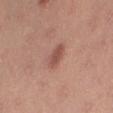This lesion was catalogued during total-body skin photography and was not selected for biopsy. A 15 mm crop from a total-body photograph taken for skin-cancer surveillance. The subject is a female aged 23 to 27. The lesion-visualizer software estimated an area of roughly 5.5 mm², an outline eccentricity of about 0.8 (0 = round, 1 = elongated), and two-axis asymmetry of about 0.2. The software also gave an average lesion color of about L≈52 a*≈24 b*≈26 (CIELAB), a lesion–skin lightness drop of about 9, and a normalized border contrast of about 6.5. The software also gave border irregularity of about 2 on a 0–10 scale, a within-lesion color-variation index near 3/10, and peripheral color asymmetry of about 1. It also reported an automated nevus-likeness rating near 70 out of 100 and lesion-presence confidence of about 100/100. Imaged with white-light lighting. Located on the right thigh. The lesion's longest dimension is about 3 mm.